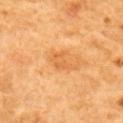follow-up=no biopsy performed (imaged during a skin exam) | image=~15 mm crop, total-body skin-cancer survey | site=the upper back | image-analysis metrics=a footprint of about 5 mm², a shape eccentricity near 0.8, and a symmetry-axis asymmetry near 0.4; roughly 8 lightness units darker than nearby skin; a detector confidence of about 100 out of 100 that the crop contains a lesion | size=≈3.5 mm | patient=male, aged around 60.